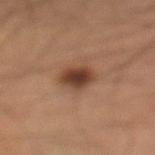<lesion>
  <biopsy_status>not biopsied; imaged during a skin examination</biopsy_status>
</lesion>